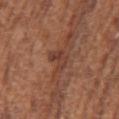The lesion was photographed on a routine skin check and not biopsied; there is no pathology result.
From the arm.
Longest diameter approximately 3 mm.
A close-up tile cropped from a whole-body skin photograph, about 15 mm across.
A female subject in their mid-70s.
This is a white-light tile.